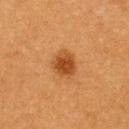A 15 mm close-up tile from a total-body photography series done for melanoma screening. Longest diameter approximately 3 mm. From the upper back. A female subject, aged approximately 55.